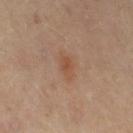Clinical impression:
Imaged during a routine full-body skin examination; the lesion was not biopsied and no histopathology is available.
Image and clinical context:
A 15 mm close-up extracted from a 3D total-body photography capture. A female subject, aged 48 to 52. Automated tile analysis of the lesion measured a footprint of about 4 mm² and two-axis asymmetry of about 0.2. About 3 mm across. The lesion is on the left thigh. Imaged with cross-polarized lighting.